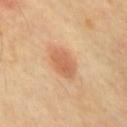Clinical summary: The lesion-visualizer software estimated an automated nevus-likeness rating near 100 out of 100 and lesion-presence confidence of about 100/100. From the chest. Cropped from a total-body skin-imaging series; the visible field is about 15 mm. The recorded lesion diameter is about 4 mm. Captured under cross-polarized illumination.